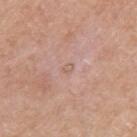{
  "biopsy_status": "not biopsied; imaged during a skin examination",
  "site": "left arm",
  "lighting": "white-light",
  "patient": {
    "sex": "male",
    "age_approx": 80
  },
  "image": {
    "source": "total-body photography crop",
    "field_of_view_mm": 15
  },
  "lesion_size": {
    "long_diameter_mm_approx": 1.5
  }
}